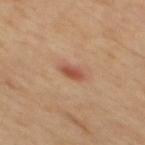Case summary:
– biopsy status — no biopsy performed (imaged during a skin exam)
– TBP lesion metrics — a mean CIELAB color near L≈49 a*≈24 b*≈30, roughly 10 lightness units darker than nearby skin, and a normalized lesion–skin contrast near 7.5; an automated nevus-likeness rating near 80 out of 100
– patient — male, aged 58 to 62
– anatomic site — the mid back
– tile lighting — cross-polarized
– acquisition — ~15 mm tile from a whole-body skin photo
– size — ≈2.5 mm